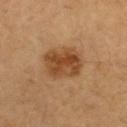follow-up — imaged on a skin check; not biopsied | diameter — about 4 mm | patient — male, aged approximately 60 | automated metrics — a footprint of about 13 mm², an outline eccentricity of about 0.45 (0 = round, 1 = elongated), and two-axis asymmetry of about 0.15; a lesion color around L≈39 a*≈19 b*≈32 in CIELAB, about 10 CIELAB-L* units darker than the surrounding skin, and a lesion-to-skin contrast of about 8.5 (normalized; higher = more distinct); a border-irregularity rating of about 2/10, a color-variation rating of about 4.5/10, and a peripheral color-asymmetry measure near 1.5; an automated nevus-likeness rating near 95 out of 100 and a detector confidence of about 100 out of 100 that the crop contains a lesion | illumination — cross-polarized | image — total-body-photography crop, ~15 mm field of view.